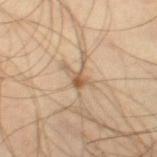Q: Was a biopsy performed?
A: imaged on a skin check; not biopsied
Q: Where on the body is the lesion?
A: the right thigh
Q: Patient demographics?
A: male, aged 43 to 47
Q: How large is the lesion?
A: ≈3.5 mm
Q: What kind of image is this?
A: ~15 mm tile from a whole-body skin photo
Q: Automated lesion metrics?
A: roughly 10 lightness units darker than nearby skin and a lesion-to-skin contrast of about 7 (normalized; higher = more distinct); a color-variation rating of about 3/10 and peripheral color asymmetry of about 1
Q: What lighting was used for the tile?
A: cross-polarized illumination